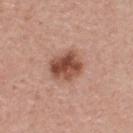Imaged during a routine full-body skin examination; the lesion was not biopsied and no histopathology is available. Cropped from a total-body skin-imaging series; the visible field is about 15 mm. A male patient about 60 years old. On the mid back.